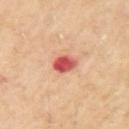Impression: The lesion was photographed on a routine skin check and not biopsied; there is no pathology result. Clinical summary: A male subject aged approximately 70. On the left upper arm. Imaged with cross-polarized lighting. A roughly 15 mm field-of-view crop from a total-body skin photograph. Automated tile analysis of the lesion measured a footprint of about 5 mm² and a shape eccentricity near 0.65. The software also gave a lesion color around L≈54 a*≈37 b*≈30 in CIELAB, roughly 17 lightness units darker than nearby skin, and a lesion-to-skin contrast of about 11 (normalized; higher = more distinct). It also reported an automated nevus-likeness rating near 0 out of 100.